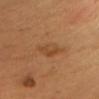<lesion>
<biopsy_status>not biopsied; imaged during a skin examination</biopsy_status>
<image>
  <source>total-body photography crop</source>
  <field_of_view_mm>15</field_of_view_mm>
</image>
<site>chest</site>
<patient>
  <sex>male</sex>
  <age_approx>65</age_approx>
</patient>
<lesion_size>
  <long_diameter_mm_approx>4.0</long_diameter_mm_approx>
</lesion_size>
</lesion>